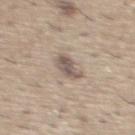Assessment: This lesion was catalogued during total-body skin photography and was not selected for biopsy. Acquisition and patient details: A male patient approximately 60 years of age. The tile uses white-light illumination. A lesion tile, about 15 mm wide, cut from a 3D total-body photograph. The lesion is located on the chest. Approximately 3 mm at its widest.